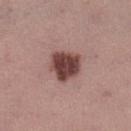Clinical impression:
Captured during whole-body skin photography for melanoma surveillance; the lesion was not biopsied.
Context:
A female patient, approximately 55 years of age. The lesion is located on the left lower leg. This image is a 15 mm lesion crop taken from a total-body photograph. An algorithmic analysis of the crop reported an automated nevus-likeness rating near 85 out of 100 and a detector confidence of about 100 out of 100 that the crop contains a lesion. Approximately 3.5 mm at its widest. Imaged with white-light lighting.